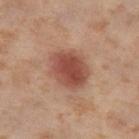Case summary:
– biopsy status: imaged on a skin check; not biopsied
– subject: female, aged 53 to 57
– automated lesion analysis: an outline eccentricity of about 0.5 (0 = round, 1 = elongated) and a shape-asymmetry score of about 0.1 (0 = symmetric); border irregularity of about 1.5 on a 0–10 scale, a within-lesion color-variation index near 4/10, and radial color variation of about 1; a nevus-likeness score of about 100/100 and a detector confidence of about 100 out of 100 that the crop contains a lesion
– anatomic site: the right thigh
– illumination: cross-polarized illumination
– diameter: ~4.5 mm (longest diameter)
– image source: ~15 mm tile from a whole-body skin photo